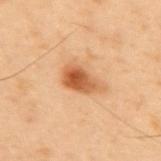The lesion was tiled from a total-body skin photograph and was not biopsied. Cropped from a whole-body photographic skin survey; the tile spans about 15 mm. Imaged with cross-polarized lighting. An algorithmic analysis of the crop reported a footprint of about 8 mm² and a symmetry-axis asymmetry near 0.3. The software also gave an average lesion color of about L≈47 a*≈22 b*≈34 (CIELAB), about 12 CIELAB-L* units darker than the surrounding skin, and a normalized lesion–skin contrast near 9. Approximately 4.5 mm at its widest. On the mid back. A male patient in their mid- to late 50s.